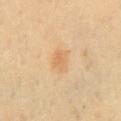Notes:
* biopsy status — no biopsy performed (imaged during a skin exam)
* lesion diameter — ~3.5 mm (longest diameter)
* acquisition — total-body-photography crop, ~15 mm field of view
* anatomic site — the mid back
* lighting — cross-polarized illumination
* patient — female, about 65 years old
* automated lesion analysis — a lesion area of about 6 mm², an eccentricity of roughly 0.75, and two-axis asymmetry of about 0.2; a nevus-likeness score of about 5/100